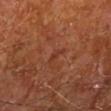<record>
<biopsy_status>not biopsied; imaged during a skin examination</biopsy_status>
<site>left lower leg</site>
<automated_metrics>
  <area_mm2_approx>2.0</area_mm2_approx>
  <eccentricity>0.95</eccentricity>
  <shape_asymmetry>0.45</shape_asymmetry>
  <cielab_L>35</cielab_L>
  <cielab_a>25</cielab_a>
  <cielab_b>30</cielab_b>
  <vs_skin_darker_L>5.0</vs_skin_darker_L>
  <vs_skin_contrast_norm>5.0</vs_skin_contrast_norm>
  <border_irregularity_0_10>5.0</border_irregularity_0_10>
  <color_variation_0_10>0.0</color_variation_0_10>
  <peripheral_color_asymmetry>0.0</peripheral_color_asymmetry>
  <nevus_likeness_0_100>0</nevus_likeness_0_100>
  <lesion_detection_confidence_0_100>100</lesion_detection_confidence_0_100>
</automated_metrics>
<lighting>cross-polarized</lighting>
<image>
  <source>total-body photography crop</source>
  <field_of_view_mm>15</field_of_view_mm>
</image>
<patient>
  <sex>male</sex>
  <age_approx>65</age_approx>
</patient>
<lesion_size>
  <long_diameter_mm_approx>2.5</long_diameter_mm_approx>
</lesion_size>
</record>